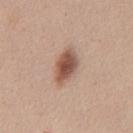Recorded during total-body skin imaging; not selected for excision or biopsy.
The lesion is on the abdomen.
The recorded lesion diameter is about 4 mm.
An algorithmic analysis of the crop reported a footprint of about 7.5 mm², a shape eccentricity near 0.8, and a symmetry-axis asymmetry near 0.2. It also reported an average lesion color of about L≈52 a*≈20 b*≈28 (CIELAB), roughly 15 lightness units darker than nearby skin, and a normalized lesion–skin contrast near 10. It also reported an automated nevus-likeness rating near 95 out of 100.
A male patient about 45 years old.
Captured under white-light illumination.
A region of skin cropped from a whole-body photographic capture, roughly 15 mm wide.